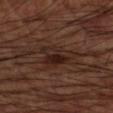{
  "biopsy_status": "not biopsied; imaged during a skin examination",
  "lesion_size": {
    "long_diameter_mm_approx": 4.5
  },
  "patient": {
    "sex": "male",
    "age_approx": 60
  },
  "site": "left upper arm",
  "automated_metrics": {
    "border_irregularity_0_10": 5.0,
    "color_variation_0_10": 5.5
  },
  "image": {
    "source": "total-body photography crop",
    "field_of_view_mm": 15
  }
}